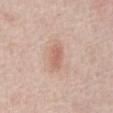Q: Was this lesion biopsied?
A: imaged on a skin check; not biopsied
Q: Where on the body is the lesion?
A: the abdomen
Q: Patient demographics?
A: male, approximately 75 years of age
Q: What is the imaging modality?
A: ~15 mm crop, total-body skin-cancer survey
Q: Automated lesion metrics?
A: a lesion color around L≈62 a*≈21 b*≈27 in CIELAB and a lesion-to-skin contrast of about 6 (normalized; higher = more distinct); a nevus-likeness score of about 30/100 and lesion-presence confidence of about 100/100
Q: What lighting was used for the tile?
A: white-light illumination
Q: Lesion size?
A: about 3 mm